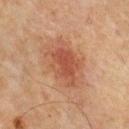Q: Was this lesion biopsied?
A: no biopsy performed (imaged during a skin exam)
Q: Lesion size?
A: about 6 mm
Q: Automated lesion metrics?
A: an area of roughly 18 mm², a shape eccentricity near 0.8, and a shape-asymmetry score of about 0.2 (0 = symmetric); border irregularity of about 3 on a 0–10 scale, internal color variation of about 4.5 on a 0–10 scale, and radial color variation of about 1.5
Q: What is the anatomic site?
A: the upper back
Q: What are the patient's age and sex?
A: male, in their mid-60s
Q: How was the tile lit?
A: cross-polarized illumination
Q: What is the imaging modality?
A: ~15 mm crop, total-body skin-cancer survey